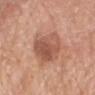• follow-up · total-body-photography surveillance lesion; no biopsy
• acquisition · 15 mm crop, total-body photography
• anatomic site · the head or neck
• size · ~5.5 mm (longest diameter)
• tile lighting · white-light illumination
• patient · male, about 80 years old